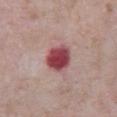{"lighting": "white-light", "site": "abdomen", "image": {"source": "total-body photography crop", "field_of_view_mm": 15}, "patient": {"sex": "male", "age_approx": 65}, "automated_metrics": {"border_irregularity_0_10": 1.0, "color_variation_0_10": 4.0, "peripheral_color_asymmetry": 1.0, "lesion_detection_confidence_0_100": 100}, "lesion_size": {"long_diameter_mm_approx": 3.5}}